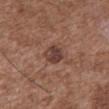Part of a total-body skin-imaging series; this lesion was reviewed on a skin check and was not flagged for biopsy.